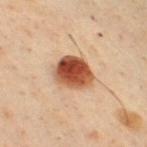  biopsy_status: not biopsied; imaged during a skin examination
  patient:
    sex: male
    age_approx: 50
  site: chest
  image:
    source: total-body photography crop
    field_of_view_mm: 15
  lighting: cross-polarized